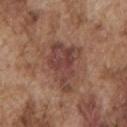Assessment: The lesion was photographed on a routine skin check and not biopsied; there is no pathology result. Context: An algorithmic analysis of the crop reported a lesion area of about 16 mm². The analysis additionally found border irregularity of about 5 on a 0–10 scale, a color-variation rating of about 4/10, and a peripheral color-asymmetry measure near 1.5. From the left upper arm. Cropped from a total-body skin-imaging series; the visible field is about 15 mm. Approximately 5.5 mm at its widest. Captured under white-light illumination. A male patient, roughly 75 years of age.